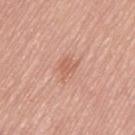A female patient aged 63–67. The tile uses white-light illumination. Cropped from a whole-body photographic skin survey; the tile spans about 15 mm. The lesion-visualizer software estimated roughly 7 lightness units darker than nearby skin and a lesion-to-skin contrast of about 5 (normalized; higher = more distinct). It also reported border irregularity of about 3 on a 0–10 scale and a color-variation rating of about 1/10. The software also gave lesion-presence confidence of about 100/100. Longest diameter approximately 2.5 mm. Located on the right thigh.